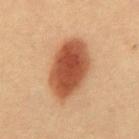Notes:
• follow-up — total-body-photography surveillance lesion; no biopsy
• image — total-body-photography crop, ~15 mm field of view
• diameter — ~7 mm (longest diameter)
• tile lighting — cross-polarized illumination
• automated lesion analysis — a footprint of about 23 mm², a shape eccentricity near 0.8, and two-axis asymmetry of about 0.1; a border-irregularity rating of about 1.5/10 and a color-variation rating of about 5/10; a nevus-likeness score of about 100/100
• patient — female, aged around 50